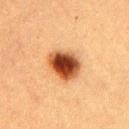{"biopsy_status": "not biopsied; imaged during a skin examination", "patient": {"sex": "male", "age_approx": 40}, "site": "right upper arm", "lighting": "cross-polarized", "image": {"source": "total-body photography crop", "field_of_view_mm": 15}}